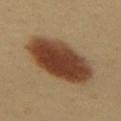follow-up — total-body-photography surveillance lesion; no biopsy
image source — 15 mm crop, total-body photography
anatomic site — the back
illumination — cross-polarized
subject — female, about 40 years old
lesion diameter — about 8.5 mm